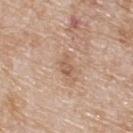Assessment: Imaged during a routine full-body skin examination; the lesion was not biopsied and no histopathology is available. Acquisition and patient details: A 15 mm close-up extracted from a 3D total-body photography capture. Located on the back. Longest diameter approximately 2.5 mm. The subject is a male approximately 80 years of age. Imaged with white-light lighting.